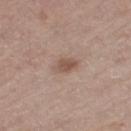Q: Was this lesion biopsied?
A: no biopsy performed (imaged during a skin exam)
Q: Lesion location?
A: the left thigh
Q: Automated lesion metrics?
A: a footprint of about 4.5 mm² and an eccentricity of roughly 0.75; a classifier nevus-likeness of about 60/100
Q: Patient demographics?
A: male, aged 53 to 57
Q: What lighting was used for the tile?
A: white-light
Q: How large is the lesion?
A: ≈3 mm
Q: What kind of image is this?
A: ~15 mm tile from a whole-body skin photo